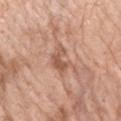tile lighting: white-light | image-analysis metrics: an average lesion color of about L≈56 a*≈22 b*≈30 (CIELAB), roughly 10 lightness units darker than nearby skin, and a normalized lesion–skin contrast near 6.5; a detector confidence of about 100 out of 100 that the crop contains a lesion | lesion size: ~3.5 mm (longest diameter) | imaging modality: 15 mm crop, total-body photography | location: the left forearm | patient: female, about 65 years old.